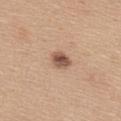Impression:
This lesion was catalogued during total-body skin photography and was not selected for biopsy.
Context:
Imaged with white-light lighting. Cropped from a total-body skin-imaging series; the visible field is about 15 mm. On the upper back. A female subject in their mid-30s.